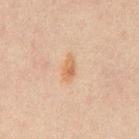Recorded during total-body skin imaging; not selected for excision or biopsy. A close-up tile cropped from a whole-body skin photograph, about 15 mm across. The lesion is located on the abdomen. A male subject, about 30 years old.